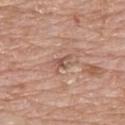{"biopsy_status": "not biopsied; imaged during a skin examination", "lighting": "white-light", "automated_metrics": {"border_irregularity_0_10": 4.5, "color_variation_0_10": 2.5, "peripheral_color_asymmetry": 0.5}, "patient": {"sex": "female", "age_approx": 65}, "lesion_size": {"long_diameter_mm_approx": 2.5}, "image": {"source": "total-body photography crop", "field_of_view_mm": 15}, "site": "front of the torso"}